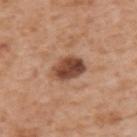  biopsy_status: not biopsied; imaged during a skin examination
  patient:
    sex: male
    age_approx: 65
  lighting: white-light
  site: back
  image:
    source: total-body photography crop
    field_of_view_mm: 15